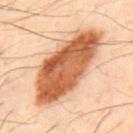No biopsy was performed on this lesion — it was imaged during a full skin examination and was not determined to be concerning. Cropped from a whole-body photographic skin survey; the tile spans about 15 mm. The lesion is located on the upper back. The lesion-visualizer software estimated a lesion area of about 41 mm², a shape eccentricity near 0.9, and two-axis asymmetry of about 0.15. And it measured a lesion–skin lightness drop of about 20 and a lesion-to-skin contrast of about 12 (normalized; higher = more distinct). This is a cross-polarized tile. A male subject aged approximately 50. About 11.5 mm across.